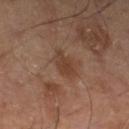Assessment:
The lesion was photographed on a routine skin check and not biopsied; there is no pathology result.
Acquisition and patient details:
A 15 mm close-up tile from a total-body photography series done for melanoma screening. The total-body-photography lesion software estimated a footprint of about 4 mm², a shape eccentricity near 0.75, and a symmetry-axis asymmetry near 0.4. And it measured a mean CIELAB color near L≈36 a*≈18 b*≈26, roughly 7 lightness units darker than nearby skin, and a lesion-to-skin contrast of about 6.5 (normalized; higher = more distinct). And it measured a nevus-likeness score of about 0/100 and lesion-presence confidence of about 100/100. From the leg. Measured at roughly 3 mm in maximum diameter. A male subject aged around 55.